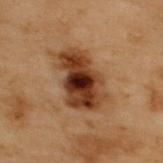Captured during whole-body skin photography for melanoma surveillance; the lesion was not biopsied. The lesion-visualizer software estimated an area of roughly 21 mm², an outline eccentricity of about 0.8 (0 = round, 1 = elongated), and two-axis asymmetry of about 0.25. The software also gave a lesion–skin lightness drop of about 14 and a normalized border contrast of about 12.5. Located on the upper back. A lesion tile, about 15 mm wide, cut from a 3D total-body photograph. A male subject aged approximately 55. The lesion's longest dimension is about 6.5 mm.